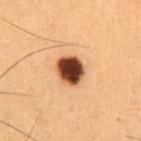Q: Was this lesion biopsied?
A: no biopsy performed (imaged during a skin exam)
Q: Automated lesion metrics?
A: a mean CIELAB color near L≈36 a*≈21 b*≈28, roughly 23 lightness units darker than nearby skin, and a lesion-to-skin contrast of about 17.5 (normalized; higher = more distinct); a nevus-likeness score of about 100/100 and lesion-presence confidence of about 100/100
Q: What is the anatomic site?
A: the abdomen
Q: Who is the patient?
A: male, approximately 50 years of age
Q: What is the imaging modality?
A: ~15 mm tile from a whole-body skin photo
Q: Illumination type?
A: cross-polarized
Q: What is the lesion's diameter?
A: ≈3.5 mm